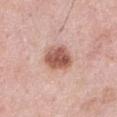| key | value |
|---|---|
| notes | catalogued during a skin exam; not biopsied |
| body site | the abdomen |
| subject | male, about 55 years old |
| acquisition | ~15 mm tile from a whole-body skin photo |